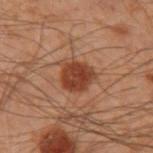<lesion>
  <biopsy_status>not biopsied; imaged during a skin examination</biopsy_status>
  <site>arm</site>
  <lesion_size>
    <long_diameter_mm_approx>4.0</long_diameter_mm_approx>
  </lesion_size>
  <lighting>cross-polarized</lighting>
  <patient>
    <sex>male</sex>
    <age_approx>50</age_approx>
  </patient>
  <image>
    <source>total-body photography crop</source>
    <field_of_view_mm>15</field_of_view_mm>
  </image>
</lesion>